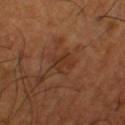Recorded during total-body skin imaging; not selected for excision or biopsy. A male patient, approximately 65 years of age. A roughly 15 mm field-of-view crop from a total-body skin photograph. The lesion is on the left lower leg.